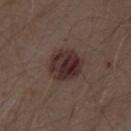biopsy status: total-body-photography surveillance lesion; no biopsy
imaging modality: 15 mm crop, total-body photography
tile lighting: white-light
anatomic site: the abdomen
automated metrics: a footprint of about 13 mm² and a shape-asymmetry score of about 0.1 (0 = symmetric); a border-irregularity index near 1/10, a within-lesion color-variation index near 6.5/10, and a peripheral color-asymmetry measure near 2.5; a classifier nevus-likeness of about 35/100
subject: male, roughly 70 years of age
lesion diameter: about 4 mm